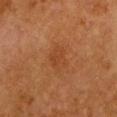Assessment:
Part of a total-body skin-imaging series; this lesion was reviewed on a skin check and was not flagged for biopsy.
Background:
The lesion's longest dimension is about 3.5 mm. A female subject in their 50s. On the chest. A 15 mm close-up extracted from a 3D total-body photography capture. Automated tile analysis of the lesion measured an area of roughly 6.5 mm², an outline eccentricity of about 0.75 (0 = round, 1 = elongated), and a shape-asymmetry score of about 0.25 (0 = symmetric). It also reported a border-irregularity rating of about 2.5/10, a within-lesion color-variation index near 2/10, and radial color variation of about 0.5. The software also gave a nevus-likeness score of about 10/100 and a lesion-detection confidence of about 100/100.